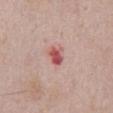Impression: The lesion was photographed on a routine skin check and not biopsied; there is no pathology result. Background: A 15 mm crop from a total-body photograph taken for skin-cancer surveillance. This is a white-light tile. The lesion is located on the abdomen. A male patient roughly 65 years of age.